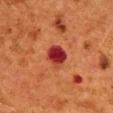The lesion was photographed on a routine skin check and not biopsied; there is no pathology result.
Cropped from a whole-body photographic skin survey; the tile spans about 15 mm.
A female subject aged approximately 50.
About 3 mm across.
Automated image analysis of the tile measured a footprint of about 6.5 mm² and a shape eccentricity near 0.4. The software also gave a mean CIELAB color near L≈31 a*≈35 b*≈27 and a normalized border contrast of about 13. The software also gave an automated nevus-likeness rating near 0 out of 100 and a lesion-detection confidence of about 100/100.
Located on the mid back.